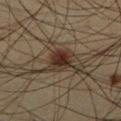Findings:
• workup — no biopsy performed (imaged during a skin exam)
• TBP lesion metrics — a footprint of about 6 mm² and two-axis asymmetry of about 0.2
• location — the right lower leg
• acquisition — ~15 mm tile from a whole-body skin photo
• patient — male, approximately 35 years of age
• diameter — ~3 mm (longest diameter)